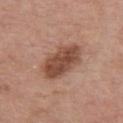Assessment:
This lesion was catalogued during total-body skin photography and was not selected for biopsy.
Background:
The tile uses white-light illumination. Measured at roughly 5.5 mm in maximum diameter. An algorithmic analysis of the crop reported a lesion area of about 14 mm². And it measured an average lesion color of about L≈47 a*≈22 b*≈28 (CIELAB) and a lesion–skin lightness drop of about 13. And it measured a border-irregularity index near 2/10, a within-lesion color-variation index near 3.5/10, and peripheral color asymmetry of about 1.5. The software also gave a nevus-likeness score of about 65/100. A male subject, aged around 60. This image is a 15 mm lesion crop taken from a total-body photograph. Located on the chest.